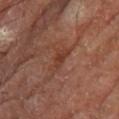workup: total-body-photography surveillance lesion; no biopsy
tile lighting: cross-polarized
subject: male, aged 68–72
anatomic site: the right thigh
lesion diameter: ~3.5 mm (longest diameter)
imaging modality: total-body-photography crop, ~15 mm field of view
automated lesion analysis: an area of roughly 5 mm², an eccentricity of roughly 0.85, and a shape-asymmetry score of about 0.4 (0 = symmetric)